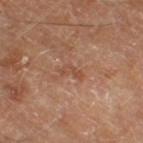This lesion was catalogued during total-body skin photography and was not selected for biopsy. The patient is a male aged approximately 70. The lesion is located on the right thigh. A lesion tile, about 15 mm wide, cut from a 3D total-body photograph.